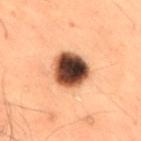Findings:
– body site: the lower back
– acquisition: total-body-photography crop, ~15 mm field of view
– tile lighting: cross-polarized illumination
– TBP lesion metrics: an average lesion color of about L≈36 a*≈19 b*≈26 (CIELAB), about 25 CIELAB-L* units darker than the surrounding skin, and a lesion-to-skin contrast of about 19 (normalized; higher = more distinct)
– patient: male, about 50 years old